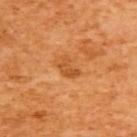The lesion was photographed on a routine skin check and not biopsied; there is no pathology result. A female subject in their mid-50s. The lesion is located on the upper back. Cropped from a total-body skin-imaging series; the visible field is about 15 mm.